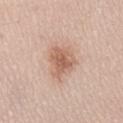– follow-up — imaged on a skin check; not biopsied
– location — the back
– image — total-body-photography crop, ~15 mm field of view
– lighting — white-light
– patient — male, aged 53–57